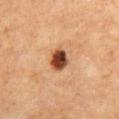workup: total-body-photography surveillance lesion; no biopsy | site: the chest | image source: 15 mm crop, total-body photography | diameter: about 3 mm | subject: female, approximately 60 years of age | lighting: cross-polarized | TBP lesion metrics: a lesion color around L≈37 a*≈21 b*≈30 in CIELAB, a lesion–skin lightness drop of about 17, and a lesion-to-skin contrast of about 13.5 (normalized; higher = more distinct).